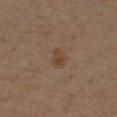The tile uses cross-polarized illumination.
Automated image analysis of the tile measured a shape eccentricity near 0.8 and a symmetry-axis asymmetry near 0.3. The analysis additionally found internal color variation of about 2 on a 0–10 scale and peripheral color asymmetry of about 1. The analysis additionally found a nevus-likeness score of about 20/100.
Measured at roughly 2.5 mm in maximum diameter.
On the right upper arm.
A region of skin cropped from a whole-body photographic capture, roughly 15 mm wide.
The subject is a male roughly 45 years of age.